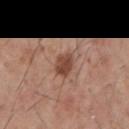Assessment:
The lesion was photographed on a routine skin check and not biopsied; there is no pathology result.
Background:
A region of skin cropped from a whole-body photographic capture, roughly 15 mm wide. A male patient aged 58 to 62. Automated image analysis of the tile measured a footprint of about 5 mm² and a shape eccentricity near 0.7. It also reported a border-irregularity rating of about 3/10 and internal color variation of about 2.5 on a 0–10 scale. It also reported a nevus-likeness score of about 90/100 and a detector confidence of about 100 out of 100 that the crop contains a lesion. Approximately 3 mm at its widest. On the mid back. Captured under white-light illumination.